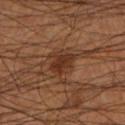Impression: Imaged during a routine full-body skin examination; the lesion was not biopsied and no histopathology is available. Context: The tile uses cross-polarized illumination. Longest diameter approximately 3.5 mm. Located on the left lower leg. The subject is a male roughly 55 years of age. A roughly 15 mm field-of-view crop from a total-body skin photograph.